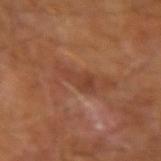Captured under cross-polarized illumination. Measured at roughly 4 mm in maximum diameter. This image is a 15 mm lesion crop taken from a total-body photograph. The subject is a male about 65 years old.